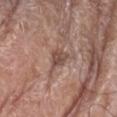TBP lesion metrics = a border-irregularity rating of about 4.5/10 and a color-variation rating of about 2.5/10; lighting = white-light illumination; subject = male, approximately 85 years of age or older; body site = the left upper arm; acquisition = ~15 mm tile from a whole-body skin photo.